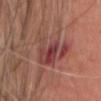workup: no biopsy performed (imaged during a skin exam)
automated metrics: a footprint of about 16 mm², an outline eccentricity of about 0.9 (0 = round, 1 = elongated), and a symmetry-axis asymmetry near 0.5; a mean CIELAB color near L≈41 a*≈25 b*≈22, about 9 CIELAB-L* units darker than the surrounding skin, and a normalized border contrast of about 8
image: ~15 mm crop, total-body skin-cancer survey
location: the head or neck
patient: male, approximately 50 years of age